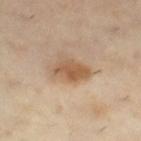<tbp_lesion>
  <biopsy_status>not biopsied; imaged during a skin examination</biopsy_status>
  <lighting>cross-polarized</lighting>
  <lesion_size>
    <long_diameter_mm_approx>4.0</long_diameter_mm_approx>
  </lesion_size>
  <site>right thigh</site>
  <automated_metrics>
    <cielab_L>57</cielab_L>
    <cielab_a>18</cielab_a>
    <cielab_b>33</cielab_b>
    <nevus_likeness_0_100>55</nevus_likeness_0_100>
  </automated_metrics>
  <patient>
    <sex>female</sex>
    <age_approx>45</age_approx>
  </patient>
  <image>
    <source>total-body photography crop</source>
    <field_of_view_mm>15</field_of_view_mm>
  </image>
</tbp_lesion>